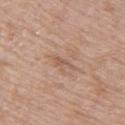The lesion was tiled from a total-body skin photograph and was not biopsied. A female patient, aged 28 to 32. This image is a 15 mm lesion crop taken from a total-body photograph. From the arm.